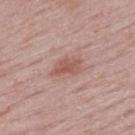follow-up = catalogued during a skin exam; not biopsied
automated metrics = an average lesion color of about L≈55 a*≈22 b*≈25 (CIELAB) and a lesion–skin lightness drop of about 9; a border-irregularity rating of about 3.5/10, a color-variation rating of about 2.5/10, and peripheral color asymmetry of about 1
lighting = white-light illumination
imaging modality = total-body-photography crop, ~15 mm field of view
subject = female, aged approximately 50
lesion size = ≈3.5 mm
anatomic site = the upper back